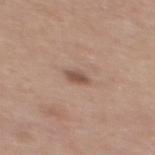{
  "biopsy_status": "not biopsied; imaged during a skin examination",
  "site": "mid back",
  "image": {
    "source": "total-body photography crop",
    "field_of_view_mm": 15
  },
  "patient": {
    "sex": "male",
    "age_approx": 30
  },
  "lighting": "white-light"
}